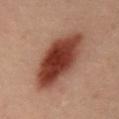biopsy_status: not biopsied; imaged during a skin examination
image:
  source: total-body photography crop
  field_of_view_mm: 15
site: abdomen
lighting: cross-polarized
patient:
  sex: male
  age_approx: 40